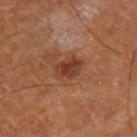Q: Was this lesion biopsied?
A: catalogued during a skin exam; not biopsied
Q: What is the anatomic site?
A: the right thigh
Q: What lighting was used for the tile?
A: cross-polarized
Q: Who is the patient?
A: male, about 65 years old
Q: Lesion size?
A: ~3.5 mm (longest diameter)
Q: What kind of image is this?
A: total-body-photography crop, ~15 mm field of view